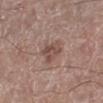image-analysis metrics: a lesion color around L≈48 a*≈18 b*≈23 in CIELAB and about 9 CIELAB-L* units darker than the surrounding skin; lesion size: about 3.5 mm; patient: male, aged approximately 65; image: total-body-photography crop, ~15 mm field of view; anatomic site: the left lower leg.